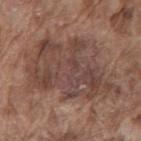Assessment: Recorded during total-body skin imaging; not selected for excision or biopsy. Context: This is a white-light tile. A male subject in their mid- to late 70s. Located on the back. A 15 mm close-up extracted from a 3D total-body photography capture.